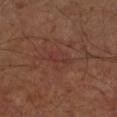Imaged during a routine full-body skin examination; the lesion was not biopsied and no histopathology is available.
A 15 mm close-up tile from a total-body photography series done for melanoma screening.
A male patient, in their mid- to late 60s.
The lesion is located on the arm.
The tile uses cross-polarized illumination.